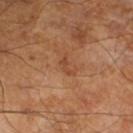This lesion was catalogued during total-body skin photography and was not selected for biopsy. A lesion tile, about 15 mm wide, cut from a 3D total-body photograph. Automated image analysis of the tile measured an area of roughly 2.5 mm², an outline eccentricity of about 0.9 (0 = round, 1 = elongated), and two-axis asymmetry of about 0.5. The analysis additionally found a normalized border contrast of about 5.5. The software also gave border irregularity of about 5.5 on a 0–10 scale, a within-lesion color-variation index near 0/10, and radial color variation of about 0. A male patient aged 63 to 67. This is a cross-polarized tile. About 2.5 mm across.